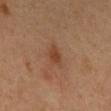<case>
  <biopsy_status>not biopsied; imaged during a skin examination</biopsy_status>
  <lighting>cross-polarized</lighting>
  <patient>
    <sex>male</sex>
    <age_approx>60</age_approx>
  </patient>
  <lesion_size>
    <long_diameter_mm_approx>3.0</long_diameter_mm_approx>
  </lesion_size>
  <site>chest</site>
  <image>
    <source>total-body photography crop</source>
    <field_of_view_mm>15</field_of_view_mm>
  </image>
</case>